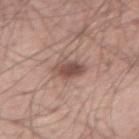<case>
<biopsy_status>not biopsied; imaged during a skin examination</biopsy_status>
<lighting>white-light</lighting>
<image>
  <source>total-body photography crop</source>
  <field_of_view_mm>15</field_of_view_mm>
</image>
<site>right thigh</site>
<patient>
  <sex>male</sex>
  <age_approx>40</age_approx>
</patient>
<automated_metrics>
  <area_mm2_approx>6.0</area_mm2_approx>
  <eccentricity>0.75</eccentricity>
  <cielab_L>50</cielab_L>
  <cielab_a>19</cielab_a>
  <cielab_b>23</cielab_b>
  <vs_skin_darker_L>12.0</vs_skin_darker_L>
  <vs_skin_contrast_norm>8.5</vs_skin_contrast_norm>
  <border_irregularity_0_10>2.5</border_irregularity_0_10>
  <peripheral_color_asymmetry>1.5</peripheral_color_asymmetry>
  <nevus_likeness_0_100>90</nevus_likeness_0_100>
</automated_metrics>
<lesion_size>
  <long_diameter_mm_approx>3.0</long_diameter_mm_approx>
</lesion_size>
</case>